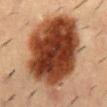Findings:
- body site — the mid back
- image source — 15 mm crop, total-body photography
- subject — male, aged 53 to 57